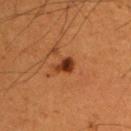Captured during whole-body skin photography for melanoma surveillance; the lesion was not biopsied. Approximately 2.5 mm at its widest. The lesion-visualizer software estimated border irregularity of about 2.5 on a 0–10 scale, a color-variation rating of about 4/10, and peripheral color asymmetry of about 1.5. And it measured a classifier nevus-likeness of about 90/100. The lesion is on the left upper arm. A male patient, roughly 50 years of age. The tile uses cross-polarized illumination. Cropped from a total-body skin-imaging series; the visible field is about 15 mm.